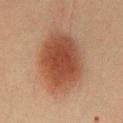No biopsy was performed on this lesion — it was imaged during a full skin examination and was not determined to be concerning.
The lesion-visualizer software estimated a lesion color around L≈39 a*≈21 b*≈27 in CIELAB and a normalized lesion–skin contrast near 9.5. The analysis additionally found an automated nevus-likeness rating near 100 out of 100.
Located on the chest.
A roughly 15 mm field-of-view crop from a total-body skin photograph.
A male subject in their 60s.
Captured under cross-polarized illumination.
About 8.5 mm across.